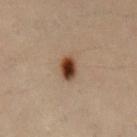Assessment:
Captured during whole-body skin photography for melanoma surveillance; the lesion was not biopsied.
Context:
Located on the mid back. About 3 mm across. Automated image analysis of the tile measured a lesion color around L≈33 a*≈16 b*≈25 in CIELAB, roughly 15 lightness units darker than nearby skin, and a normalized lesion–skin contrast near 13. A male subject aged around 30. A close-up tile cropped from a whole-body skin photograph, about 15 mm across.